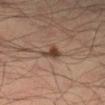Impression: No biopsy was performed on this lesion — it was imaged during a full skin examination and was not determined to be concerning. Clinical summary: The lesion's longest dimension is about 3 mm. The lesion is located on the right lower leg. This is a cross-polarized tile. A male patient about 35 years old. A region of skin cropped from a whole-body photographic capture, roughly 15 mm wide.